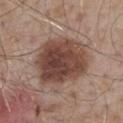Impression: Captured during whole-body skin photography for melanoma surveillance; the lesion was not biopsied. Image and clinical context: The recorded lesion diameter is about 6.5 mm. A 15 mm close-up extracted from a 3D total-body photography capture. Located on the mid back. An algorithmic analysis of the crop reported a mean CIELAB color near L≈43 a*≈19 b*≈24, roughly 14 lightness units darker than nearby skin, and a normalized border contrast of about 11. It also reported an automated nevus-likeness rating near 45 out of 100 and a detector confidence of about 100 out of 100 that the crop contains a lesion. A male subject roughly 55 years of age. Imaged with white-light lighting.